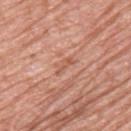follow-up=imaged on a skin check; not biopsied
subject=male, in their 60s
site=the upper back
image=15 mm crop, total-body photography